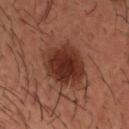  biopsy_status: not biopsied; imaged during a skin examination
  site: head or neck
  image:
    source: total-body photography crop
    field_of_view_mm: 15
  lighting: cross-polarized
  patient:
    sex: male
    age_approx: 35
  automated_metrics:
    area_mm2_approx: 20.0
    eccentricity: 0.4
    shape_asymmetry: 0.15
    cielab_L: 31
    cielab_a: 23
    cielab_b: 26
    vs_skin_contrast_norm: 11.0
  lesion_size:
    long_diameter_mm_approx: 5.5